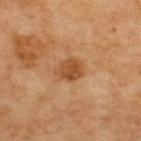Part of a total-body skin-imaging series; this lesion was reviewed on a skin check and was not flagged for biopsy. From the upper back. Imaged with cross-polarized lighting. A 15 mm close-up tile from a total-body photography series done for melanoma screening. Longest diameter approximately 3 mm.